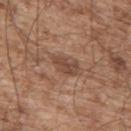Imaged during a routine full-body skin examination; the lesion was not biopsied and no histopathology is available.
An algorithmic analysis of the crop reported a lesion area of about 5.5 mm², an outline eccentricity of about 0.8 (0 = round, 1 = elongated), and a shape-asymmetry score of about 0.3 (0 = symmetric). The analysis additionally found an average lesion color of about L≈46 a*≈19 b*≈27 (CIELAB), about 9 CIELAB-L* units darker than the surrounding skin, and a normalized lesion–skin contrast near 7. And it measured a border-irregularity rating of about 3.5/10, a within-lesion color-variation index near 1.5/10, and a peripheral color-asymmetry measure near 0.5. And it measured a lesion-detection confidence of about 100/100.
The recorded lesion diameter is about 3.5 mm.
On the back.
Cropped from a total-body skin-imaging series; the visible field is about 15 mm.
Imaged with white-light lighting.
A male subject aged 53 to 57.